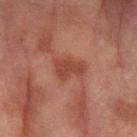Part of a total-body skin-imaging series; this lesion was reviewed on a skin check and was not flagged for biopsy. Captured under cross-polarized illumination. From the left forearm. This image is a 15 mm lesion crop taken from a total-body photograph. A male patient, aged 73–77.